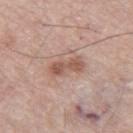The lesion was photographed on a routine skin check and not biopsied; there is no pathology result. Located on the right thigh. An algorithmic analysis of the crop reported a lesion area of about 6 mm², an eccentricity of roughly 0.9, and a shape-asymmetry score of about 0.2 (0 = symmetric). It also reported a border-irregularity index near 3/10 and a within-lesion color-variation index near 2.5/10. A male subject about 55 years old. Cropped from a whole-body photographic skin survey; the tile spans about 15 mm.